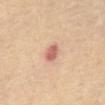Notes:
– workup: imaged on a skin check; not biopsied
– body site: the abdomen
– automated lesion analysis: an average lesion color of about L≈62 a*≈25 b*≈28 (CIELAB), roughly 12 lightness units darker than nearby skin, and a lesion-to-skin contrast of about 8 (normalized; higher = more distinct); a border-irregularity rating of about 1.5/10, internal color variation of about 3 on a 0–10 scale, and radial color variation of about 1; a nevus-likeness score of about 5/100 and lesion-presence confidence of about 100/100
– patient: female, aged 63–67
– lesion diameter: about 3 mm
– image: total-body-photography crop, ~15 mm field of view
– illumination: cross-polarized illumination